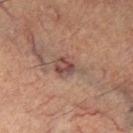<case>
<biopsy_status>not biopsied; imaged during a skin examination</biopsy_status>
<automated_metrics>
  <vs_skin_darker_L>9.0</vs_skin_darker_L>
  <border_irregularity_0_10>3.0</border_irregularity_0_10>
  <color_variation_0_10>5.0</color_variation_0_10>
  <peripheral_color_asymmetry>1.5</peripheral_color_asymmetry>
</automated_metrics>
<lesion_size>
  <long_diameter_mm_approx>4.0</long_diameter_mm_approx>
</lesion_size>
<patient>
  <sex>male</sex>
  <age_approx>60</age_approx>
</patient>
<site>left thigh</site>
<image>
  <source>total-body photography crop</source>
  <field_of_view_mm>15</field_of_view_mm>
</image>
<lighting>cross-polarized</lighting>
</case>